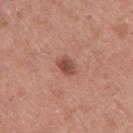{"biopsy_status": "not biopsied; imaged during a skin examination", "automated_metrics": {"area_mm2_approx": 3.5, "eccentricity": 0.75, "shape_asymmetry": 0.25, "border_irregularity_0_10": 2.0, "peripheral_color_asymmetry": 0.5}, "lighting": "white-light", "patient": {"sex": "female", "age_approx": 40}, "lesion_size": {"long_diameter_mm_approx": 2.5}, "image": {"source": "total-body photography crop", "field_of_view_mm": 15}, "site": "right upper arm"}